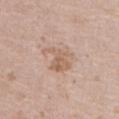An algorithmic analysis of the crop reported a footprint of about 9 mm² and two-axis asymmetry of about 0.4. The software also gave border irregularity of about 5 on a 0–10 scale and a color-variation rating of about 4/10. It also reported a classifier nevus-likeness of about 5/100 and lesion-presence confidence of about 100/100. A 15 mm close-up tile from a total-body photography series done for melanoma screening. A female patient aged around 75. The tile uses white-light illumination. The lesion is located on the abdomen. The recorded lesion diameter is about 4 mm.